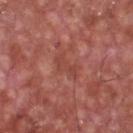Assessment:
Recorded during total-body skin imaging; not selected for excision or biopsy.